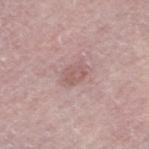No biopsy was performed on this lesion — it was imaged during a full skin examination and was not determined to be concerning.